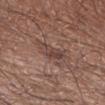<tbp_lesion>
  <biopsy_status>not biopsied; imaged during a skin examination</biopsy_status>
</tbp_lesion>